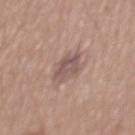Q: What is the lesion's diameter?
A: ~3.5 mm (longest diameter)
Q: Illumination type?
A: white-light
Q: What is the anatomic site?
A: the mid back
Q: Automated lesion metrics?
A: an average lesion color of about L≈53 a*≈17 b*≈21 (CIELAB), roughly 9 lightness units darker than nearby skin, and a lesion-to-skin contrast of about 7 (normalized; higher = more distinct)
Q: How was this image acquired?
A: 15 mm crop, total-body photography
Q: Who is the patient?
A: male, aged 63–67